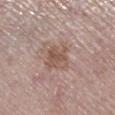Case summary:
* lighting — white-light
* image — ~15 mm crop, total-body skin-cancer survey
* lesion size — ≈3 mm
* body site — the right lower leg
* subject — female, in their mid- to late 60s